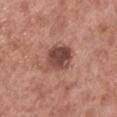No biopsy was performed on this lesion — it was imaged during a full skin examination and was not determined to be concerning.
A male subject, aged approximately 55.
Imaged with white-light lighting.
The lesion's longest dimension is about 3.5 mm.
A close-up tile cropped from a whole-body skin photograph, about 15 mm across.
The lesion is located on the right lower leg.